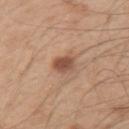Case summary:
• patient · male, about 50 years old
• lesion size · ≈2.5 mm
• automated lesion analysis · an average lesion color of about L≈51 a*≈21 b*≈29 (CIELAB), a lesion–skin lightness drop of about 12, and a normalized lesion–skin contrast near 8.5; a color-variation rating of about 3/10 and a peripheral color-asymmetry measure near 1; a detector confidence of about 100 out of 100 that the crop contains a lesion
• tile lighting · white-light illumination
• image source · 15 mm crop, total-body photography
• site · the left upper arm